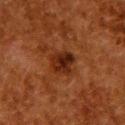Findings:
- workup: total-body-photography surveillance lesion; no biopsy
- image-analysis metrics: a shape eccentricity near 0.3; a within-lesion color-variation index near 6/10 and a peripheral color-asymmetry measure near 2; a classifier nevus-likeness of about 85/100 and lesion-presence confidence of about 100/100
- image source: 15 mm crop, total-body photography
- illumination: cross-polarized
- body site: the upper back
- diameter: ≈3.5 mm
- subject: female, approximately 50 years of age